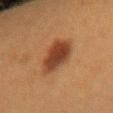– workup · total-body-photography surveillance lesion; no biopsy
– image source · ~15 mm crop, total-body skin-cancer survey
– anatomic site · the right forearm
– subject · female, roughly 40 years of age